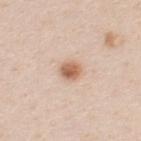On the back. This is a white-light tile. A male patient aged around 35. Approximately 2.5 mm at its widest. A close-up tile cropped from a whole-body skin photograph, about 15 mm across. Automated tile analysis of the lesion measured a lesion area of about 5 mm² and two-axis asymmetry of about 0.2.